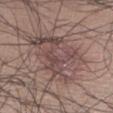biopsy_status: not biopsied; imaged during a skin examination
patient:
  sex: male
  age_approx: 65
lighting: white-light
site: right lower leg
image:
  source: total-body photography crop
  field_of_view_mm: 15